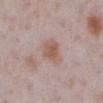Located on the right lower leg. A female patient, roughly 30 years of age. Measured at roughly 3.5 mm in maximum diameter. Cropped from a total-body skin-imaging series; the visible field is about 15 mm. Captured under white-light illumination.